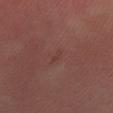site: arm
image:
  source: total-body photography crop
  field_of_view_mm: 15
patient:
  sex: male
  age_approx: 30